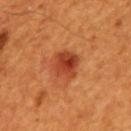{
  "image": {
    "source": "total-body photography crop",
    "field_of_view_mm": 15
  },
  "lesion_size": {
    "long_diameter_mm_approx": 3.5
  },
  "site": "back",
  "patient": {
    "sex": "male",
    "age_approx": 60
  },
  "lighting": "cross-polarized"
}